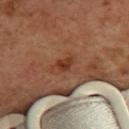Case summary:
* workup — total-body-photography surveillance lesion; no biopsy
* subject — female, aged 53–57
* image-analysis metrics — a mean CIELAB color near L≈39 a*≈25 b*≈33, a lesion–skin lightness drop of about 9, and a normalized border contrast of about 8; radial color variation of about 1; a nevus-likeness score of about 20/100 and a detector confidence of about 100 out of 100 that the crop contains a lesion
* anatomic site — the back
* tile lighting — cross-polarized
* lesion size — ~3 mm (longest diameter)
* image — 15 mm crop, total-body photography